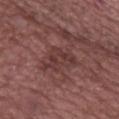{"biopsy_status": "not biopsied; imaged during a skin examination", "lesion_size": {"long_diameter_mm_approx": 4.5}, "patient": {"sex": "female", "age_approx": 75}, "automated_metrics": {"cielab_L": 36, "cielab_a": 21, "cielab_b": 19, "vs_skin_darker_L": 7.0, "vs_skin_contrast_norm": 6.0, "border_irregularity_0_10": 5.5, "color_variation_0_10": 2.5, "peripheral_color_asymmetry": 1.0}, "site": "back", "image": {"source": "total-body photography crop", "field_of_view_mm": 15}, "lighting": "white-light"}